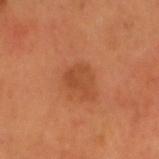Q: Where on the body is the lesion?
A: the head or neck
Q: What did automated image analysis measure?
A: a classifier nevus-likeness of about 10/100 and lesion-presence confidence of about 100/100
Q: Illumination type?
A: cross-polarized illumination
Q: What kind of image is this?
A: ~15 mm tile from a whole-body skin photo
Q: Patient demographics?
A: male, roughly 55 years of age